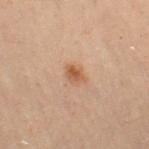* biopsy status: imaged on a skin check; not biopsied
* patient: female, aged 28 to 32
* tile lighting: cross-polarized
* imaging modality: 15 mm crop, total-body photography
* body site: the left thigh
* automated lesion analysis: a shape eccentricity near 0.65; a mean CIELAB color near L≈45 a*≈17 b*≈29 and roughly 8 lightness units darker than nearby skin; a nevus-likeness score of about 75/100 and lesion-presence confidence of about 100/100